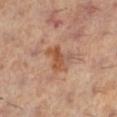  biopsy_status: not biopsied; imaged during a skin examination
  automated_metrics:
    area_mm2_approx: 6.0
    eccentricity: 0.7
  lighting: cross-polarized
  patient:
    sex: female
    age_approx: 60
  lesion_size:
    long_diameter_mm_approx: 3.5
  site: left lower leg
  image:
    source: total-body photography crop
    field_of_view_mm: 15